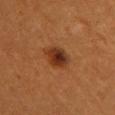No biopsy was performed on this lesion — it was imaged during a full skin examination and was not determined to be concerning.
A close-up tile cropped from a whole-body skin photograph, about 15 mm across.
A female patient, aged approximately 55.
An algorithmic analysis of the crop reported a lesion color around L≈29 a*≈21 b*≈30 in CIELAB, roughly 10 lightness units darker than nearby skin, and a normalized border contrast of about 10. The software also gave a border-irregularity index near 1.5/10, a color-variation rating of about 6.5/10, and a peripheral color-asymmetry measure near 2.
Located on the chest.
The recorded lesion diameter is about 3.5 mm.